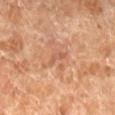biopsy status: no biopsy performed (imaged during a skin exam)
image source: ~15 mm tile from a whole-body skin photo
diameter: ≈3 mm
subject: female, roughly 70 years of age
body site: the left lower leg
automated metrics: a lesion area of about 2.5 mm², a shape eccentricity near 0.9, and a symmetry-axis asymmetry near 0.6; a mean CIELAB color near L≈56 a*≈23 b*≈32, a lesion–skin lightness drop of about 7, and a normalized lesion–skin contrast near 5; a border-irregularity index near 6/10, internal color variation of about 0 on a 0–10 scale, and peripheral color asymmetry of about 0; an automated nevus-likeness rating near 0 out of 100
illumination: cross-polarized illumination